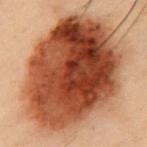subject = male, approximately 55 years of age | lesion size = ≈13.5 mm | tile lighting = cross-polarized | acquisition = total-body-photography crop, ~15 mm field of view | site = the chest.